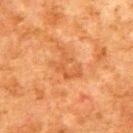Part of a total-body skin-imaging series; this lesion was reviewed on a skin check and was not flagged for biopsy.